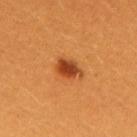Imaged during a routine full-body skin examination; the lesion was not biopsied and no histopathology is available. A region of skin cropped from a whole-body photographic capture, roughly 15 mm wide. Automated image analysis of the tile measured an average lesion color of about L≈42 a*≈29 b*≈41 (CIELAB). The software also gave a border-irregularity index near 2.5/10 and a color-variation rating of about 4.5/10. A female subject approximately 30 years of age. The lesion is located on the back. Imaged with cross-polarized lighting.